A male patient aged 63 to 67, located on the upper back, about 2.5 mm across, this is a white-light tile, this image is a 15 mm lesion crop taken from a total-body photograph.
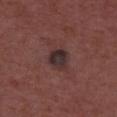The lesion was biopsied, and histopathology showed an atypical melanocytic neoplasm, classified as an indeterminate (borderline) lesion.